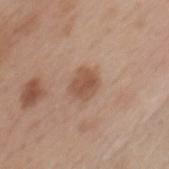Q: Is there a histopathology result?
A: no biopsy performed (imaged during a skin exam)
Q: Patient demographics?
A: female, approximately 75 years of age
Q: What is the anatomic site?
A: the chest
Q: What is the imaging modality?
A: total-body-photography crop, ~15 mm field of view